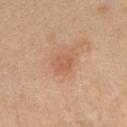notes: total-body-photography surveillance lesion; no biopsy
subject: female, aged approximately 40
TBP lesion metrics: an eccentricity of roughly 0.55 and two-axis asymmetry of about 0.2; a mean CIELAB color near L≈59 a*≈23 b*≈33, roughly 7 lightness units darker than nearby skin, and a normalized border contrast of about 4.5; internal color variation of about 2 on a 0–10 scale and peripheral color asymmetry of about 0.5
image source: total-body-photography crop, ~15 mm field of view
size: about 2.5 mm
body site: the left upper arm
tile lighting: white-light